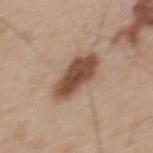{
  "biopsy_status": "not biopsied; imaged during a skin examination",
  "automated_metrics": {
    "border_irregularity_0_10": 2.5,
    "color_variation_0_10": 4.0,
    "peripheral_color_asymmetry": 1.5
  },
  "lesion_size": {
    "long_diameter_mm_approx": 5.5
  },
  "lighting": "white-light",
  "image": {
    "source": "total-body photography crop",
    "field_of_view_mm": 15
  },
  "site": "back",
  "patient": {
    "sex": "male",
    "age_approx": 65
  }
}